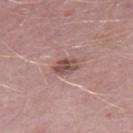lesion diameter: ≈3 mm | anatomic site: the leg | tile lighting: white-light | subject: male, about 50 years old | imaging modality: 15 mm crop, total-body photography.